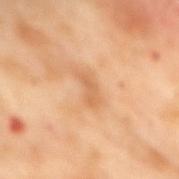workup: no biopsy performed (imaged during a skin exam) | image source: ~15 mm crop, total-body skin-cancer survey | site: the back | patient: female, roughly 55 years of age | tile lighting: cross-polarized illumination | TBP lesion metrics: a lesion color around L≈65 a*≈24 b*≈41 in CIELAB and a normalized lesion–skin contrast near 5.5; a classifier nevus-likeness of about 0/100.